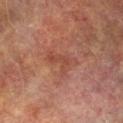| feature | finding |
|---|---|
| workup | total-body-photography surveillance lesion; no biopsy |
| patient | male, aged approximately 75 |
| anatomic site | the leg |
| imaging modality | 15 mm crop, total-body photography |
| tile lighting | cross-polarized |